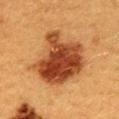No biopsy was performed on this lesion — it was imaged during a full skin examination and was not determined to be concerning. From the mid back. Measured at roughly 7 mm in maximum diameter. A close-up tile cropped from a whole-body skin photograph, about 15 mm across. A female subject aged 38–42.